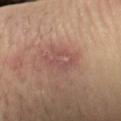biopsy status: no biopsy performed (imaged during a skin exam) | image source: ~15 mm crop, total-body skin-cancer survey | tile lighting: cross-polarized | TBP lesion metrics: an average lesion color of about L≈50 a*≈22 b*≈23 (CIELAB) and a lesion–skin lightness drop of about 6; border irregularity of about 4 on a 0–10 scale, a color-variation rating of about 3.5/10, and radial color variation of about 1.5; an automated nevus-likeness rating near 0 out of 100 and lesion-presence confidence of about 100/100 | subject: male, in their 40s | site: the left forearm | lesion diameter: ≈4 mm.